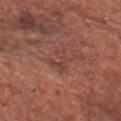lesion size = ≈3 mm | lighting = white-light illumination | patient = male, in their mid-60s | image = 15 mm crop, total-body photography | image-analysis metrics = a footprint of about 4.5 mm², a shape eccentricity near 0.65, and two-axis asymmetry of about 0.5 | body site = the head or neck.